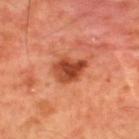| field | value |
|---|---|
| lesion size | about 3.5 mm |
| location | the upper back |
| patient | male, aged approximately 60 |
| image | ~15 mm crop, total-body skin-cancer survey |
| tile lighting | cross-polarized |